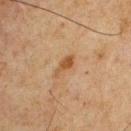Assessment:
Captured during whole-body skin photography for melanoma surveillance; the lesion was not biopsied.
Image and clinical context:
From the chest. A male patient, in their mid- to late 70s. Measured at roughly 3.5 mm in maximum diameter. A 15 mm crop from a total-body photograph taken for skin-cancer surveillance.